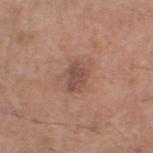Recorded during total-body skin imaging; not selected for excision or biopsy. Measured at roughly 3 mm in maximum diameter. A 15 mm crop from a total-body photograph taken for skin-cancer surveillance. Automated tile analysis of the lesion measured roughly 9 lightness units darker than nearby skin and a normalized lesion–skin contrast near 7. The analysis additionally found a border-irregularity rating of about 2/10, a within-lesion color-variation index near 2/10, and peripheral color asymmetry of about 1. And it measured a classifier nevus-likeness of about 5/100 and lesion-presence confidence of about 100/100. The lesion is located on the left lower leg. A male patient about 60 years old.